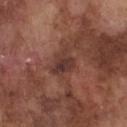The lesion was photographed on a routine skin check and not biopsied; there is no pathology result. The lesion-visualizer software estimated a lesion color around L≈36 a*≈21 b*≈23 in CIELAB, about 9 CIELAB-L* units darker than the surrounding skin, and a normalized lesion–skin contrast near 8. It also reported a border-irregularity rating of about 4/10, a within-lesion color-variation index near 4/10, and peripheral color asymmetry of about 1. The analysis additionally found a nevus-likeness score of about 0/100 and a lesion-detection confidence of about 100/100. Captured under white-light illumination. A region of skin cropped from a whole-body photographic capture, roughly 15 mm wide. A male patient, in their mid- to late 70s. About 3.5 mm across. On the chest.